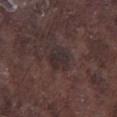notes: catalogued during a skin exam; not biopsied
lesion size: ≈3.5 mm
site: the leg
acquisition: 15 mm crop, total-body photography
illumination: white-light
patient: male, aged around 75
automated lesion analysis: an area of roughly 7 mm², an eccentricity of roughly 0.5, and a symmetry-axis asymmetry near 0.25; a lesion color around L≈28 a*≈12 b*≈13 in CIELAB and a normalized lesion–skin contrast near 6.5; a border-irregularity index near 2.5/10, internal color variation of about 3.5 on a 0–10 scale, and a peripheral color-asymmetry measure near 1.5